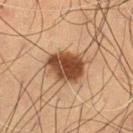Clinical impression: This lesion was catalogued during total-body skin photography and was not selected for biopsy. Context: The lesion is located on the left thigh. The subject is a male aged 48–52. This image is a 15 mm lesion crop taken from a total-body photograph. About 4.5 mm across. Imaged with cross-polarized lighting.